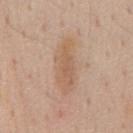site: chest
lesion_size:
  long_diameter_mm_approx: 6.5
image:
  source: total-body photography crop
  field_of_view_mm: 15
automated_metrics:
  area_mm2_approx: 13.0
  eccentricity: 0.9
  shape_asymmetry: 0.25
  cielab_L: 60
  cielab_a: 18
  cielab_b: 31
  vs_skin_darker_L: 8.0
  vs_skin_contrast_norm: 6.0
patient:
  sex: male
  age_approx: 55
lighting: white-light